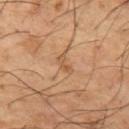Impression: Recorded during total-body skin imaging; not selected for excision or biopsy. Background: On the left thigh. A close-up tile cropped from a whole-body skin photograph, about 15 mm across. Imaged with cross-polarized lighting. A male patient about 65 years old. The recorded lesion diameter is about 3 mm.